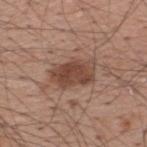The lesion was photographed on a routine skin check and not biopsied; there is no pathology result. From the upper back. Imaged with white-light lighting. Approximately 5 mm at its widest. Cropped from a whole-body photographic skin survey; the tile spans about 15 mm. A male patient in their 60s. The lesion-visualizer software estimated roughly 12 lightness units darker than nearby skin and a normalized border contrast of about 9.